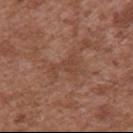biopsy status: no biopsy performed (imaged during a skin exam) | automated lesion analysis: an area of roughly 4.5 mm², an eccentricity of roughly 0.8, and a shape-asymmetry score of about 0.65 (0 = symmetric); an average lesion color of about L≈43 a*≈22 b*≈28 (CIELAB), a lesion–skin lightness drop of about 5, and a normalized lesion–skin contrast near 4.5 | patient: male, about 45 years old | image: ~15 mm crop, total-body skin-cancer survey | lesion diameter: ≈3.5 mm | body site: the upper back.